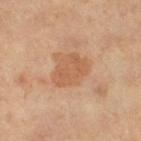This lesion was catalogued during total-body skin photography and was not selected for biopsy. From the left thigh. Approximately 4 mm at its widest. This is a cross-polarized tile. A lesion tile, about 15 mm wide, cut from a 3D total-body photograph. A female subject in their mid- to late 60s.